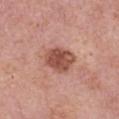The lesion was tiled from a total-body skin photograph and was not biopsied.
A male patient, aged 73 to 77.
Imaged with white-light lighting.
Longest diameter approximately 4 mm.
From the right upper arm.
Automated image analysis of the tile measured a footprint of about 10 mm², an eccentricity of roughly 0.6, and a shape-asymmetry score of about 0.2 (0 = symmetric).
A 15 mm close-up extracted from a 3D total-body photography capture.